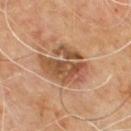Q: Was this lesion biopsied?
A: total-body-photography surveillance lesion; no biopsy
Q: How was this image acquired?
A: 15 mm crop, total-body photography
Q: Patient demographics?
A: male, aged 58–62
Q: Lesion location?
A: the upper back